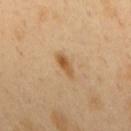Impression: The lesion was tiled from a total-body skin photograph and was not biopsied. Acquisition and patient details: Imaged with cross-polarized lighting. A male patient about 50 years old. An algorithmic analysis of the crop reported a lesion–skin lightness drop of about 10 and a lesion-to-skin contrast of about 8 (normalized; higher = more distinct). And it measured an automated nevus-likeness rating near 80 out of 100 and lesion-presence confidence of about 100/100. Located on the back. Longest diameter approximately 3 mm. A roughly 15 mm field-of-view crop from a total-body skin photograph.